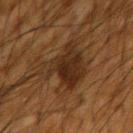Impression: The lesion was photographed on a routine skin check and not biopsied; there is no pathology result. Acquisition and patient details: Measured at roughly 6 mm in maximum diameter. The lesion is located on the left upper arm. A close-up tile cropped from a whole-body skin photograph, about 15 mm across. The patient is a male in their mid-60s. The tile uses cross-polarized illumination.